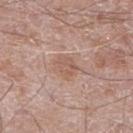Clinical impression: Recorded during total-body skin imaging; not selected for excision or biopsy. Image and clinical context: The recorded lesion diameter is about 3 mm. An algorithmic analysis of the crop reported a footprint of about 5.5 mm², a shape eccentricity near 0.6, and a symmetry-axis asymmetry near 0.25. The software also gave about 7 CIELAB-L* units darker than the surrounding skin and a lesion-to-skin contrast of about 5.5 (normalized; higher = more distinct). A male subject, approximately 60 years of age. The tile uses white-light illumination. The lesion is located on the leg. Cropped from a total-body skin-imaging series; the visible field is about 15 mm.